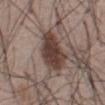Clinical impression: Recorded during total-body skin imaging; not selected for excision or biopsy.